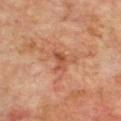This lesion was catalogued during total-body skin photography and was not selected for biopsy. Automated tile analysis of the lesion measured a classifier nevus-likeness of about 0/100. A male patient roughly 70 years of age. This is a cross-polarized tile. The lesion's longest dimension is about 2.5 mm. From the front of the torso. A lesion tile, about 15 mm wide, cut from a 3D total-body photograph.